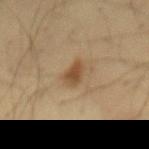Captured during whole-body skin photography for melanoma surveillance; the lesion was not biopsied. About 3 mm across. Imaged with cross-polarized lighting. The lesion is on the mid back. Cropped from a total-body skin-imaging series; the visible field is about 15 mm. The patient is a male about 35 years old.